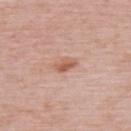biopsy status: total-body-photography surveillance lesion; no biopsy
patient: male, aged 63–67
body site: the upper back
image source: ~15 mm crop, total-body skin-cancer survey